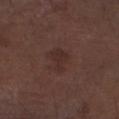notes: imaged on a skin check; not biopsied
patient: male, approximately 75 years of age
automated metrics: border irregularity of about 4.5 on a 0–10 scale, a color-variation rating of about 2/10, and radial color variation of about 0.5
lesion diameter: ~3.5 mm (longest diameter)
imaging modality: total-body-photography crop, ~15 mm field of view
anatomic site: the right forearm